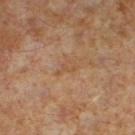Background: The recorded lesion diameter is about 2.5 mm. The lesion is on the left lower leg. A male patient aged approximately 60. Imaged with cross-polarized lighting. A roughly 15 mm field-of-view crop from a total-body skin photograph.